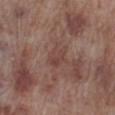biopsy_status: not biopsied; imaged during a skin examination